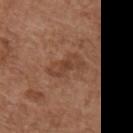Assessment: Recorded during total-body skin imaging; not selected for excision or biopsy. Clinical summary: The patient is a female in their mid-70s. A region of skin cropped from a whole-body photographic capture, roughly 15 mm wide. From the chest.